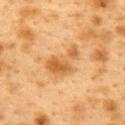{"biopsy_status": "not biopsied; imaged during a skin examination", "patient": {"sex": "female", "age_approx": 40}, "lighting": "cross-polarized", "site": "back", "image": {"source": "total-body photography crop", "field_of_view_mm": 15}}